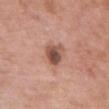Impression: The lesion was photographed on a routine skin check and not biopsied; there is no pathology result. Clinical summary: From the front of the torso. A female patient, aged around 70. A 15 mm crop from a total-body photograph taken for skin-cancer surveillance. An algorithmic analysis of the crop reported an area of roughly 6 mm², an outline eccentricity of about 0.5 (0 = round, 1 = elongated), and a shape-asymmetry score of about 0.3 (0 = symmetric). It also reported an automated nevus-likeness rating near 35 out of 100.